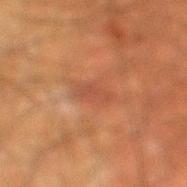- imaging modality: total-body-photography crop, ~15 mm field of view
- automated lesion analysis: a border-irregularity rating of about 3/10, a color-variation rating of about 2/10, and radial color variation of about 0.5
- patient: male, aged 48 to 52
- anatomic site: the leg
- tile lighting: cross-polarized